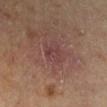On the left lower leg. A male subject, roughly 65 years of age. Captured under cross-polarized illumination. Cropped from a total-body skin-imaging series; the visible field is about 15 mm.